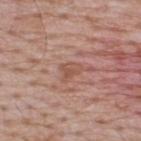Automated image analysis of the tile measured a mean CIELAB color near L≈53 a*≈22 b*≈28. The analysis additionally found a border-irregularity index near 4/10, a color-variation rating of about 2/10, and radial color variation of about 0.5. The software also gave a lesion-detection confidence of about 100/100.
Captured under white-light illumination.
Cropped from a total-body skin-imaging series; the visible field is about 15 mm.
A male subject roughly 65 years of age.
About 3 mm across.
Located on the upper back.